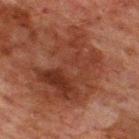- workup: imaged on a skin check; not biopsied
- image: ~15 mm tile from a whole-body skin photo
- subject: male, about 60 years old
- body site: the upper back
- automated metrics: an area of roughly 37 mm², an eccentricity of roughly 0.7, and two-axis asymmetry of about 0.35; an average lesion color of about L≈28 a*≈20 b*≈24 (CIELAB) and a normalized border contrast of about 7
- size: ~9 mm (longest diameter)